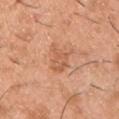subject: male, aged approximately 40; size: ≈4 mm; site: the chest; illumination: white-light illumination; image: ~15 mm crop, total-body skin-cancer survey.